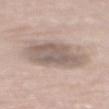The lesion was tiled from a total-body skin photograph and was not biopsied.
A male patient, about 85 years old or older.
Automated image analysis of the tile measured an outline eccentricity of about 0.65 (0 = round, 1 = elongated) and two-axis asymmetry of about 0.2. The analysis additionally found a nevus-likeness score of about 5/100 and a detector confidence of about 90 out of 100 that the crop contains a lesion.
From the upper back.
Cropped from a whole-body photographic skin survey; the tile spans about 15 mm.